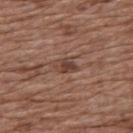workup: no biopsy performed (imaged during a skin exam)
body site: the back
automated metrics: a footprint of about 3.5 mm², an eccentricity of roughly 0.65, and a shape-asymmetry score of about 0.3 (0 = symmetric); a border-irregularity index near 3/10 and a color-variation rating of about 2.5/10; a nevus-likeness score of about 5/100 and a lesion-detection confidence of about 100/100
size: ≈2.5 mm
subject: female, in their mid-70s
acquisition: 15 mm crop, total-body photography